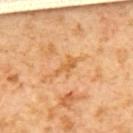Clinical impression:
Part of a total-body skin-imaging series; this lesion was reviewed on a skin check and was not flagged for biopsy.
Clinical summary:
A female patient approximately 55 years of age. The tile uses cross-polarized illumination. A region of skin cropped from a whole-body photographic capture, roughly 15 mm wide. On the upper back. Measured at roughly 3 mm in maximum diameter.